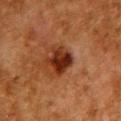{
  "biopsy_status": "not biopsied; imaged during a skin examination",
  "image": {
    "source": "total-body photography crop",
    "field_of_view_mm": 15
  },
  "site": "chest",
  "patient": {
    "sex": "female",
    "age_approx": 50
  }
}